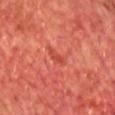Impression:
Imaged during a routine full-body skin examination; the lesion was not biopsied and no histopathology is available.
Context:
From the chest. An algorithmic analysis of the crop reported a border-irregularity rating of about 3/10 and a color-variation rating of about 0/10. And it measured an automated nevus-likeness rating near 0 out of 100 and a lesion-detection confidence of about 95/100. Cropped from a whole-body photographic skin survey; the tile spans about 15 mm. A male subject aged 63 to 67. The recorded lesion diameter is about 2.5 mm. Imaged with cross-polarized lighting.